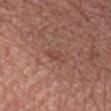{"biopsy_status": "not biopsied; imaged during a skin examination", "image": {"source": "total-body photography crop", "field_of_view_mm": 15}, "patient": {"sex": "male", "age_approx": 55}}